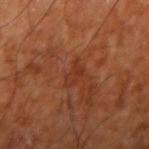The lesion was photographed on a routine skin check and not biopsied; there is no pathology result. Approximately 3 mm at its widest. A 15 mm crop from a total-body photograph taken for skin-cancer surveillance. Captured under cross-polarized illumination. An algorithmic analysis of the crop reported a lesion–skin lightness drop of about 5 and a normalized border contrast of about 5. And it measured border irregularity of about 3 on a 0–10 scale, a color-variation rating of about 2.5/10, and a peripheral color-asymmetry measure near 1. And it measured a nevus-likeness score of about 0/100 and lesion-presence confidence of about 100/100. From the right upper arm. The patient is approximately 65 years of age.